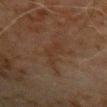Part of a total-body skin-imaging series; this lesion was reviewed on a skin check and was not flagged for biopsy. Approximately 5 mm at its widest. A roughly 15 mm field-of-view crop from a total-body skin photograph. Imaged with cross-polarized lighting. A male subject aged approximately 70. The lesion is on the right upper arm.